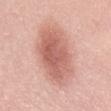notes: total-body-photography surveillance lesion; no biopsy
diameter: ≈7.5 mm
lighting: white-light illumination
patient: female, in their mid- to late 50s
acquisition: ~15 mm tile from a whole-body skin photo
anatomic site: the lower back